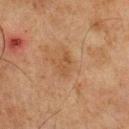Clinical impression: Part of a total-body skin-imaging series; this lesion was reviewed on a skin check and was not flagged for biopsy. Image and clinical context: Approximately 3 mm at its widest. Imaged with cross-polarized lighting. The lesion is located on the chest. This image is a 15 mm lesion crop taken from a total-body photograph. A male patient, about 75 years old.